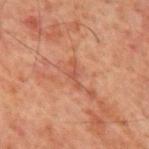{"biopsy_status": "not biopsied; imaged during a skin examination", "lesion_size": {"long_diameter_mm_approx": 3.5}, "patient": {"sex": "male", "age_approx": 60}, "site": "mid back", "image": {"source": "total-body photography crop", "field_of_view_mm": 15}}